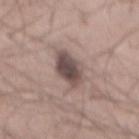From the mid back.
Cropped from a whole-body photographic skin survey; the tile spans about 15 mm.
This is a white-light tile.
An algorithmic analysis of the crop reported a footprint of about 10 mm² and two-axis asymmetry of about 0.15. The software also gave an average lesion color of about L≈48 a*≈14 b*≈18 (CIELAB) and a lesion-to-skin contrast of about 10.5 (normalized; higher = more distinct).
Longest diameter approximately 5 mm.
A male subject, aged 48–52.